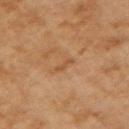biopsy status: catalogued during a skin exam; not biopsied
lesion diameter: ~2.5 mm (longest diameter)
TBP lesion metrics: a lesion area of about 1.5 mm², a shape eccentricity near 0.95, and two-axis asymmetry of about 0.45; internal color variation of about 0 on a 0–10 scale and a peripheral color-asymmetry measure near 0; an automated nevus-likeness rating near 0 out of 100
anatomic site: the left upper arm
tile lighting: cross-polarized
image: 15 mm crop, total-body photography
patient: female, aged 58 to 62